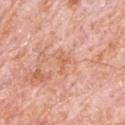<tbp_lesion>
<biopsy_status>not biopsied; imaged during a skin examination</biopsy_status>
<lighting>white-light</lighting>
<lesion_size>
  <long_diameter_mm_approx>2.5</long_diameter_mm_approx>
</lesion_size>
<site>back</site>
<image>
  <source>total-body photography crop</source>
  <field_of_view_mm>15</field_of_view_mm>
</image>
<patient>
  <sex>male</sex>
  <age_approx>80</age_approx>
</patient>
<automated_metrics>
  <cielab_L>63</cielab_L>
  <cielab_a>25</cielab_a>
  <cielab_b>34</cielab_b>
  <vs_skin_darker_L>7.0</vs_skin_darker_L>
  <nevus_likeness_0_100>0</nevus_likeness_0_100>
  <lesion_detection_confidence_0_100>100</lesion_detection_confidence_0_100>
</automated_metrics>
</tbp_lesion>